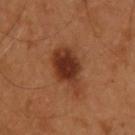Assessment: The lesion was photographed on a routine skin check and not biopsied; there is no pathology result. Image and clinical context: Located on the upper back. The patient is a male roughly 55 years of age. A region of skin cropped from a whole-body photographic capture, roughly 15 mm wide.